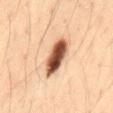Q: Is there a histopathology result?
A: total-body-photography surveillance lesion; no biopsy
Q: Where on the body is the lesion?
A: the lower back
Q: Patient demographics?
A: male, in their mid- to late 30s
Q: How was the tile lit?
A: cross-polarized
Q: Lesion size?
A: ~5 mm (longest diameter)
Q: Automated lesion metrics?
A: an average lesion color of about L≈50 a*≈22 b*≈31 (CIELAB) and a normalized border contrast of about 14.5; a border-irregularity rating of about 1.5/10, a color-variation rating of about 9/10, and peripheral color asymmetry of about 3; a classifier nevus-likeness of about 100/100 and lesion-presence confidence of about 100/100
Q: What kind of image is this?
A: ~15 mm crop, total-body skin-cancer survey